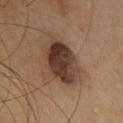Findings:
• workup · total-body-photography surveillance lesion; no biopsy
• tile lighting · cross-polarized illumination
• site · the upper back
• image-analysis metrics · an area of roughly 18 mm², an eccentricity of roughly 0.8, and two-axis asymmetry of about 0.15; a border-irregularity index near 1.5/10, internal color variation of about 8 on a 0–10 scale, and peripheral color asymmetry of about 3; a classifier nevus-likeness of about 25/100
• lesion size · ~6 mm (longest diameter)
• subject · female, roughly 40 years of age
• image source · ~15 mm tile from a whole-body skin photo